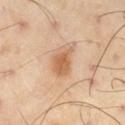biopsy status — total-body-photography surveillance lesion; no biopsy | lighting — cross-polarized illumination | image source — total-body-photography crop, ~15 mm field of view | location — the right thigh | TBP lesion metrics — an area of roughly 6.5 mm² and an outline eccentricity of about 0.75 (0 = round, 1 = elongated); a border-irregularity rating of about 3/10, a within-lesion color-variation index near 2.5/10, and a peripheral color-asymmetry measure near 0.5 | subject — male, in their mid-50s.